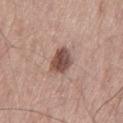follow-up = total-body-photography surveillance lesion; no biopsy
anatomic site = the left thigh
image source = 15 mm crop, total-body photography
lighting = white-light illumination
patient = male, aged 53 to 57